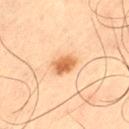The lesion was photographed on a routine skin check and not biopsied; there is no pathology result. Approximately 3 mm at its widest. A 15 mm close-up tile from a total-body photography series done for melanoma screening. Captured under cross-polarized illumination. The subject is a male in their mid-60s. On the left thigh.